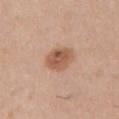  biopsy_status: not biopsied; imaged during a skin examination
  lighting: white-light
  site: chest
  image:
    source: total-body photography crop
    field_of_view_mm: 15
  lesion_size:
    long_diameter_mm_approx: 3.5
  patient:
    sex: male
    age_approx: 40